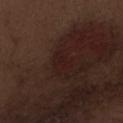TBP lesion metrics: a footprint of about 3.5 mm² and a shape eccentricity near 0.75; a normalized lesion–skin contrast near 5.5; an automated nevus-likeness rating near 0 out of 100
acquisition: ~15 mm tile from a whole-body skin photo
body site: the abdomen
size: ~2.5 mm (longest diameter)
illumination: white-light
subject: male, aged 68–72